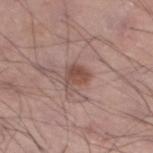<case>
<biopsy_status>not biopsied; imaged during a skin examination</biopsy_status>
<lesion_size>
  <long_diameter_mm_approx>3.0</long_diameter_mm_approx>
</lesion_size>
<site>left lower leg</site>
<patient>
  <sex>male</sex>
  <age_approx>50</age_approx>
</patient>
<image>
  <source>total-body photography crop</source>
  <field_of_view_mm>15</field_of_view_mm>
</image>
<lighting>white-light</lighting>
</case>